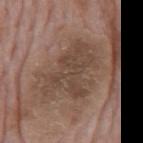The lesion was photographed on a routine skin check and not biopsied; there is no pathology result. The recorded lesion diameter is about 7.5 mm. A male subject, roughly 75 years of age. The lesion is on the back. A region of skin cropped from a whole-body photographic capture, roughly 15 mm wide. The tile uses white-light illumination.